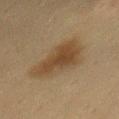Clinical impression:
Imaged during a routine full-body skin examination; the lesion was not biopsied and no histopathology is available.
Acquisition and patient details:
This is a cross-polarized tile. The lesion is located on the chest. A lesion tile, about 15 mm wide, cut from a 3D total-body photograph. Longest diameter approximately 5.5 mm. A female patient, aged 38 to 42.